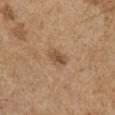biopsy_status: not biopsied; imaged during a skin examination
patient:
  sex: male
  age_approx: 65
lighting: white-light
image:
  source: total-body photography crop
  field_of_view_mm: 15
lesion_size:
  long_diameter_mm_approx: 2.5
site: right upper arm
automated_metrics:
  eccentricity: 0.75
  shape_asymmetry: 0.3
  nevus_likeness_0_100: 70
  lesion_detection_confidence_0_100: 100